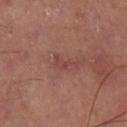Cropped from a total-body skin-imaging series; the visible field is about 15 mm.
The lesion is located on the right thigh.
Automated tile analysis of the lesion measured a footprint of about 3 mm², a shape eccentricity near 0.9, and a shape-asymmetry score of about 0.55 (0 = symmetric). It also reported a border-irregularity rating of about 6.5/10, internal color variation of about 0 on a 0–10 scale, and a peripheral color-asymmetry measure near 0.
The tile uses cross-polarized illumination.
About 3.5 mm across.